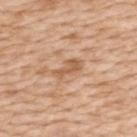{
  "biopsy_status": "not biopsied; imaged during a skin examination",
  "patient": {
    "sex": "female",
    "age_approx": 45
  },
  "site": "upper back",
  "lighting": "white-light",
  "lesion_size": {
    "long_diameter_mm_approx": 3.5
  },
  "automated_metrics": {
    "eccentricity": 0.9,
    "border_irregularity_0_10": 4.5,
    "color_variation_0_10": 2.0
  },
  "image": {
    "source": "total-body photography crop",
    "field_of_view_mm": 15
  }
}